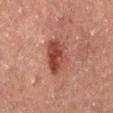Impression:
Imaged during a routine full-body skin examination; the lesion was not biopsied and no histopathology is available.
Background:
The lesion's longest dimension is about 5 mm. A male patient in their 60s. A region of skin cropped from a whole-body photographic capture, roughly 15 mm wide. The lesion is located on the mid back.